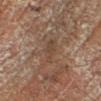The lesion was photographed on a routine skin check and not biopsied; there is no pathology result.
A male patient aged approximately 65.
From the right lower leg.
A roughly 15 mm field-of-view crop from a total-body skin photograph.
An algorithmic analysis of the crop reported a shape eccentricity near 0.9 and a shape-asymmetry score of about 0.3 (0 = symmetric). The software also gave an average lesion color of about L≈43 a*≈16 b*≈26 (CIELAB), about 6 CIELAB-L* units darker than the surrounding skin, and a lesion-to-skin contrast of about 5 (normalized; higher = more distinct).
This is a cross-polarized tile.